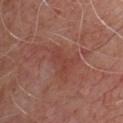follow-up: no biopsy performed (imaged during a skin exam) | automated metrics: a symmetry-axis asymmetry near 0.4; a border-irregularity rating of about 5/10, internal color variation of about 0.5 on a 0–10 scale, and radial color variation of about 0; lesion-presence confidence of about 100/100 | location: the chest | illumination: cross-polarized illumination | acquisition: total-body-photography crop, ~15 mm field of view | subject: male, approximately 50 years of age.